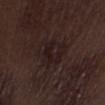  biopsy_status: not biopsied; imaged during a skin examination
  image:
    source: total-body photography crop
    field_of_view_mm: 15
  lesion_size:
    long_diameter_mm_approx: 5.0
  lighting: white-light
  site: right thigh
  patient:
    sex: male
    age_approx: 70
  automated_metrics:
    cielab_L: 18
    cielab_a: 13
    cielab_b: 13
    vs_skin_contrast_norm: 7.0